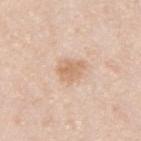Clinical impression:
Part of a total-body skin-imaging series; this lesion was reviewed on a skin check and was not flagged for biopsy.
Background:
A male patient, roughly 35 years of age. Cropped from a whole-body photographic skin survey; the tile spans about 15 mm. From the upper back. This is a white-light tile. About 3.5 mm across.